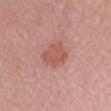Clinical impression:
Captured during whole-body skin photography for melanoma surveillance; the lesion was not biopsied.
Clinical summary:
Located on the chest. The patient is a female approximately 50 years of age. A 15 mm crop from a total-body photograph taken for skin-cancer surveillance.